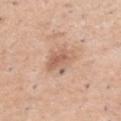{
  "biopsy_status": "not biopsied; imaged during a skin examination",
  "site": "right upper arm",
  "lighting": "white-light",
  "patient": {
    "sex": "male",
    "age_approx": 50
  },
  "automated_metrics": {
    "area_mm2_approx": 7.5,
    "eccentricity": 0.35,
    "shape_asymmetry": 0.3,
    "cielab_L": 61,
    "cielab_a": 20,
    "cielab_b": 30,
    "vs_skin_darker_L": 10.0,
    "vs_skin_contrast_norm": 6.5,
    "border_irregularity_0_10": 3.5,
    "color_variation_0_10": 3.5,
    "peripheral_color_asymmetry": 1.0,
    "nevus_likeness_0_100": 40
  },
  "lesion_size": {
    "long_diameter_mm_approx": 3.0
  },
  "image": {
    "source": "total-body photography crop",
    "field_of_view_mm": 15
  }
}